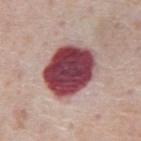Q: Is there a histopathology result?
A: catalogued during a skin exam; not biopsied